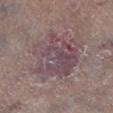No biopsy was performed on this lesion — it was imaged during a full skin examination and was not determined to be concerning. A male patient, aged around 85 or older. The lesion is located on the right lower leg. Cropped from a whole-body photographic skin survey; the tile spans about 15 mm.